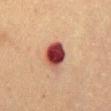The lesion was photographed on a routine skin check and not biopsied; there is no pathology result. Imaged with cross-polarized lighting. A 15 mm close-up extracted from a 3D total-body photography capture. From the abdomen. Measured at roughly 3.5 mm in maximum diameter. The subject is a male aged approximately 75. The lesion-visualizer software estimated a footprint of about 9.5 mm², an outline eccentricity of about 0.5 (0 = round, 1 = elongated), and a shape-asymmetry score of about 0.2 (0 = symmetric). It also reported an average lesion color of about L≈36 a*≈26 b*≈23 (CIELAB), roughly 19 lightness units darker than nearby skin, and a normalized lesion–skin contrast near 15. The software also gave internal color variation of about 8.5 on a 0–10 scale and a peripheral color-asymmetry measure near 2.5.